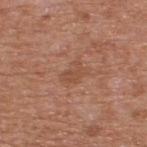Part of a total-body skin-imaging series; this lesion was reviewed on a skin check and was not flagged for biopsy. The lesion-visualizer software estimated an area of roughly 4.5 mm², a shape eccentricity near 0.75, and a shape-asymmetry score of about 0.35 (0 = symmetric). The software also gave a mean CIELAB color near L≈49 a*≈22 b*≈31, about 6 CIELAB-L* units darker than the surrounding skin, and a normalized border contrast of about 5. A male patient aged 63 to 67. Captured under white-light illumination. A close-up tile cropped from a whole-body skin photograph, about 15 mm across. The lesion's longest dimension is about 3 mm. Located on the upper back.